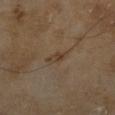Captured during whole-body skin photography for melanoma surveillance; the lesion was not biopsied.
A male subject about 65 years old.
A region of skin cropped from a whole-body photographic capture, roughly 15 mm wide.
On the right lower leg.
The lesion-visualizer software estimated an eccentricity of roughly 0.9 and a shape-asymmetry score of about 0.15 (0 = symmetric). The analysis additionally found a mean CIELAB color near L≈39 a*≈14 b*≈27, a lesion–skin lightness drop of about 6, and a lesion-to-skin contrast of about 6 (normalized; higher = more distinct). And it measured a border-irregularity index near 2/10, internal color variation of about 3.5 on a 0–10 scale, and radial color variation of about 1.5. And it measured a nevus-likeness score of about 0/100.
This is a cross-polarized tile.
Approximately 3 mm at its widest.